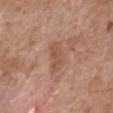Impression: Recorded during total-body skin imaging; not selected for excision or biopsy. Background: A roughly 15 mm field-of-view crop from a total-body skin photograph. The lesion is on the right forearm. Automated tile analysis of the lesion measured a lesion area of about 5 mm² and an outline eccentricity of about 0.9 (0 = round, 1 = elongated). The software also gave a lesion color around L≈52 a*≈21 b*≈29 in CIELAB. The software also gave a nevus-likeness score of about 0/100. The lesion's longest dimension is about 3.5 mm. The patient is a female aged approximately 75.